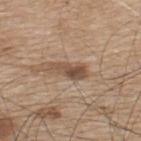No biopsy was performed on this lesion — it was imaged during a full skin examination and was not determined to be concerning.
The lesion is located on the upper back.
This image is a 15 mm lesion crop taken from a total-body photograph.
A male subject aged 73–77.
The tile uses white-light illumination.